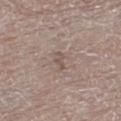biopsy status: imaged on a skin check; not biopsied
patient: male, approximately 80 years of age
image-analysis metrics: a lesion area of about 2.5 mm², an outline eccentricity of about 0.9 (0 = round, 1 = elongated), and a shape-asymmetry score of about 0.3 (0 = symmetric); an automated nevus-likeness rating near 0 out of 100
tile lighting: white-light illumination
image: 15 mm crop, total-body photography
diameter: about 2.5 mm
site: the left thigh